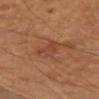No biopsy was performed on this lesion — it was imaged during a full skin examination and was not determined to be concerning. Automated tile analysis of the lesion measured a lesion color around L≈39 a*≈21 b*≈28 in CIELAB and a lesion-to-skin contrast of about 5 (normalized; higher = more distinct). The patient is a male roughly 55 years of age. A region of skin cropped from a whole-body photographic capture, roughly 15 mm wide. This is a cross-polarized tile. On the left upper arm. Approximately 4 mm at its widest.